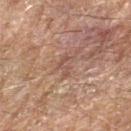Q: Is there a histopathology result?
A: imaged on a skin check; not biopsied
Q: What did automated image analysis measure?
A: a mean CIELAB color near L≈37 a*≈15 b*≈20, a lesion–skin lightness drop of about 5, and a normalized lesion–skin contrast near 5
Q: What is the anatomic site?
A: the left forearm
Q: What are the patient's age and sex?
A: male, aged around 60
Q: What lighting was used for the tile?
A: cross-polarized
Q: How was this image acquired?
A: ~15 mm crop, total-body skin-cancer survey